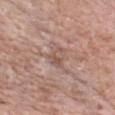  lighting: white-light
  lesion_size:
    long_diameter_mm_approx: 3.0
  site: chest
  patient:
    sex: female
    age_approx: 75
  automated_metrics:
    nevus_likeness_0_100: 0
    lesion_detection_confidence_0_100: 65
  image:
    source: total-body photography crop
    field_of_view_mm: 15